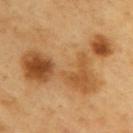Imaged during a routine full-body skin examination; the lesion was not biopsied and no histopathology is available.
The lesion is on the upper back.
A male patient, roughly 60 years of age.
A roughly 15 mm field-of-view crop from a total-body skin photograph.
Measured at roughly 10.5 mm in maximum diameter.
The tile uses cross-polarized illumination.